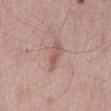Assessment:
This lesion was catalogued during total-body skin photography and was not selected for biopsy.
Acquisition and patient details:
The subject is a male aged around 65. A roughly 15 mm field-of-view crop from a total-body skin photograph. The lesion is located on the back.